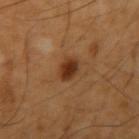lighting: cross-polarized illumination
anatomic site: the right upper arm
imaging modality: total-body-photography crop, ~15 mm field of view
lesion size: ≈3 mm
subject: male, aged approximately 65